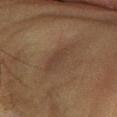Findings:
• biopsy status — imaged on a skin check; not biopsied
• lesion size — about 5 mm
• subject — male, aged 58–62
• automated metrics — a border-irregularity index near 3.5/10, a within-lesion color-variation index near 2/10, and peripheral color asymmetry of about 0.5
• image source — ~15 mm crop, total-body skin-cancer survey
• site — the leg